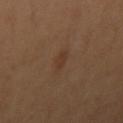Acquisition and patient details:
A female patient aged 38 to 42. This image is a 15 mm lesion crop taken from a total-body photograph. Automated image analysis of the tile measured a lesion-detection confidence of about 100/100. The lesion is located on the arm. Approximately 2.5 mm at its widest.